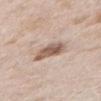Q: Was this lesion biopsied?
A: no biopsy performed (imaged during a skin exam)
Q: What are the patient's age and sex?
A: male, aged around 40
Q: How was the tile lit?
A: white-light illumination
Q: Automated lesion metrics?
A: a footprint of about 7.5 mm², an outline eccentricity of about 0.7 (0 = round, 1 = elongated), and a shape-asymmetry score of about 0.25 (0 = symmetric); roughly 14 lightness units darker than nearby skin and a lesion-to-skin contrast of about 9 (normalized; higher = more distinct); a border-irregularity index near 2.5/10, internal color variation of about 4.5 on a 0–10 scale, and peripheral color asymmetry of about 1.5; a nevus-likeness score of about 85/100 and a lesion-detection confidence of about 100/100
Q: What kind of image is this?
A: 15 mm crop, total-body photography
Q: What is the anatomic site?
A: the arm